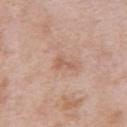Captured during whole-body skin photography for melanoma surveillance; the lesion was not biopsied.
The lesion is located on the left upper arm.
The recorded lesion diameter is about 2.5 mm.
A male subject, aged approximately 55.
Imaged with white-light lighting.
Cropped from a total-body skin-imaging series; the visible field is about 15 mm.